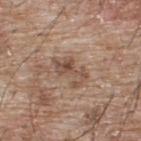Captured during whole-body skin photography for melanoma surveillance; the lesion was not biopsied. A male patient, aged around 65. Automated image analysis of the tile measured an average lesion color of about L≈50 a*≈18 b*≈27 (CIELAB). And it measured border irregularity of about 9 on a 0–10 scale and a peripheral color-asymmetry measure near 1. A region of skin cropped from a whole-body photographic capture, roughly 15 mm wide. Measured at roughly 4 mm in maximum diameter. On the upper back. Imaged with white-light lighting.